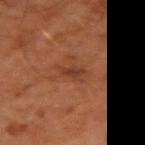This lesion was catalogued during total-body skin photography and was not selected for biopsy. A male patient, aged 63–67. On the right upper arm. An algorithmic analysis of the crop reported a lesion color around L≈36 a*≈24 b*≈31 in CIELAB, roughly 7 lightness units darker than nearby skin, and a normalized border contrast of about 6.5. The software also gave a border-irregularity rating of about 3.5/10, a color-variation rating of about 0.5/10, and radial color variation of about 0. The analysis additionally found an automated nevus-likeness rating near 0 out of 100 and a detector confidence of about 100 out of 100 that the crop contains a lesion. A 15 mm close-up extracted from a 3D total-body photography capture.